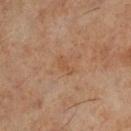Impression:
Imaged during a routine full-body skin examination; the lesion was not biopsied and no histopathology is available.